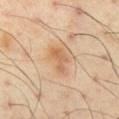This lesion was catalogued during total-body skin photography and was not selected for biopsy. A 15 mm close-up extracted from a 3D total-body photography capture. On the left thigh. Measured at roughly 4 mm in maximum diameter. The subject is a male aged 53 to 57. The tile uses cross-polarized illumination.